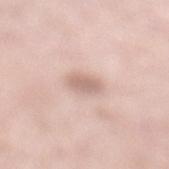Recorded during total-body skin imaging; not selected for excision or biopsy.
Captured under white-light illumination.
An algorithmic analysis of the crop reported an area of roughly 4.5 mm² and a shape eccentricity near 0.6. And it measured about 10 CIELAB-L* units darker than the surrounding skin and a lesion-to-skin contrast of about 6.5 (normalized; higher = more distinct). The analysis additionally found an automated nevus-likeness rating near 65 out of 100 and lesion-presence confidence of about 100/100.
From the left lower leg.
A 15 mm crop from a total-body photograph taken for skin-cancer surveillance.
The patient is a female approximately 50 years of age.